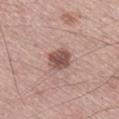Recorded during total-body skin imaging; not selected for excision or biopsy.
Located on the right lower leg.
A male subject roughly 50 years of age.
This image is a 15 mm lesion crop taken from a total-body photograph.